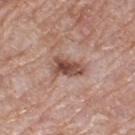Impression: The lesion was photographed on a routine skin check and not biopsied; there is no pathology result. Background: Longest diameter approximately 4 mm. On the leg. The patient is a female approximately 70 years of age. An algorithmic analysis of the crop reported a footprint of about 8 mm² and an outline eccentricity of about 0.85 (0 = round, 1 = elongated). It also reported roughly 12 lightness units darker than nearby skin and a lesion-to-skin contrast of about 8.5 (normalized; higher = more distinct). A roughly 15 mm field-of-view crop from a total-body skin photograph.